A lesion tile, about 15 mm wide, cut from a 3D total-body photograph. A male patient, approximately 30 years of age. Located on the chest:
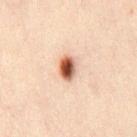Conclusion:
On excision, pathology confirmed a benign skin lesion: dysplastic (Clark) nevus.